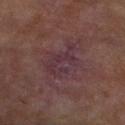This lesion was catalogued during total-body skin photography and was not selected for biopsy.
The lesion is on the left lower leg.
The total-body-photography lesion software estimated a lesion area of about 10 mm² and two-axis asymmetry of about 0.35. The analysis additionally found a mean CIELAB color near L≈28 a*≈17 b*≈11 and a normalized lesion–skin contrast near 7.5. It also reported a lesion-detection confidence of about 60/100.
This is a cross-polarized tile.
The recorded lesion diameter is about 5 mm.
A 15 mm close-up tile from a total-body photography series done for melanoma screening.
The patient is a female aged approximately 60.